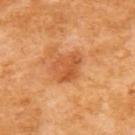Imaged during a routine full-body skin examination; the lesion was not biopsied and no histopathology is available.
From the upper back.
The recorded lesion diameter is about 3.5 mm.
A female patient, in their mid- to late 50s.
The total-body-photography lesion software estimated a lesion area of about 8.5 mm², an eccentricity of roughly 0.55, and a shape-asymmetry score of about 0.3 (0 = symmetric). The software also gave a border-irregularity rating of about 3/10, internal color variation of about 4 on a 0–10 scale, and a peripheral color-asymmetry measure near 1.
A close-up tile cropped from a whole-body skin photograph, about 15 mm across.